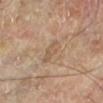{"biopsy_status": "not biopsied; imaged during a skin examination", "image": {"source": "total-body photography crop", "field_of_view_mm": 15}, "site": "left lower leg", "automated_metrics": {"nevus_likeness_0_100": 0, "lesion_detection_confidence_0_100": 95}, "patient": {"sex": "male", "age_approx": 70}, "lesion_size": {"long_diameter_mm_approx": 3.0}, "lighting": "cross-polarized"}